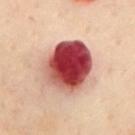Case summary:
* workup: imaged on a skin check; not biopsied
* patient: female, aged 58–62
* image: ~15 mm tile from a whole-body skin photo
* site: the chest
* TBP lesion metrics: an eccentricity of roughly 0.45 and a shape-asymmetry score of about 0.15 (0 = symmetric); a border-irregularity rating of about 1.5/10; a nevus-likeness score of about 35/100 and a detector confidence of about 100 out of 100 that the crop contains a lesion